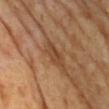Imaged during a routine full-body skin examination; the lesion was not biopsied and no histopathology is available. Approximately 3 mm at its widest. A female patient aged 68 to 72. Cropped from a total-body skin-imaging series; the visible field is about 15 mm. Imaged with cross-polarized lighting. The lesion is located on the upper back. The total-body-photography lesion software estimated a lesion area of about 4 mm², an eccentricity of roughly 0.9, and a shape-asymmetry score of about 0.3 (0 = symmetric). It also reported a classifier nevus-likeness of about 0/100 and a lesion-detection confidence of about 60/100.